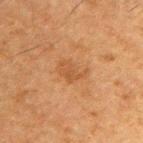| key | value |
|---|---|
| notes | total-body-photography surveillance lesion; no biopsy |
| illumination | cross-polarized |
| image | total-body-photography crop, ~15 mm field of view |
| automated metrics | a footprint of about 6 mm², an eccentricity of roughly 0.8, and a symmetry-axis asymmetry near 0.35; border irregularity of about 4 on a 0–10 scale and a color-variation rating of about 2/10; a lesion-detection confidence of about 100/100 |
| patient | male, aged around 50 |
| body site | the upper back |